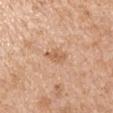biopsy status: imaged on a skin check; not biopsied | image source: ~15 mm tile from a whole-body skin photo | patient: male, approximately 65 years of age | body site: the left upper arm | automated metrics: a mean CIELAB color near L≈61 a*≈22 b*≈34, a lesion–skin lightness drop of about 9, and a lesion-to-skin contrast of about 6 (normalized; higher = more distinct); a nevus-likeness score of about 5/100 and lesion-presence confidence of about 100/100.